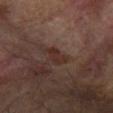The lesion is on the right forearm.
The subject is a male aged 63–67.
This is a cross-polarized tile.
A 15 mm close-up tile from a total-body photography series done for melanoma screening.